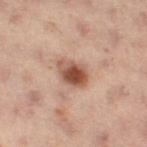Context: A female subject, about 55 years old. Cropped from a whole-body photographic skin survey; the tile spans about 15 mm. This is a cross-polarized tile. The lesion is on the left leg. An algorithmic analysis of the crop reported a mean CIELAB color near L≈43 a*≈19 b*≈25, a lesion–skin lightness drop of about 13, and a lesion-to-skin contrast of about 10 (normalized; higher = more distinct). It also reported border irregularity of about 2 on a 0–10 scale and a peripheral color-asymmetry measure near 2. And it measured a nevus-likeness score of about 95/100 and lesion-presence confidence of about 100/100. Measured at roughly 3.5 mm in maximum diameter.